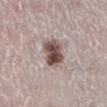Recorded during total-body skin imaging; not selected for excision or biopsy. From the right lower leg. Automated image analysis of the tile measured a lesion–skin lightness drop of about 17 and a normalized border contrast of about 12. The analysis additionally found a border-irregularity index near 2/10, a color-variation rating of about 6.5/10, and radial color variation of about 2.5. A female subject, approximately 50 years of age. A close-up tile cropped from a whole-body skin photograph, about 15 mm across. This is a white-light tile.